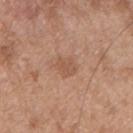{"biopsy_status": "not biopsied; imaged during a skin examination", "automated_metrics": {"area_mm2_approx": 4.0, "eccentricity": 0.6, "shape_asymmetry": 0.3, "cielab_L": 54, "cielab_a": 21, "cielab_b": 31, "vs_skin_darker_L": 7.0, "vs_skin_contrast_norm": 5.5, "color_variation_0_10": 2.0, "peripheral_color_asymmetry": 1.0, "nevus_likeness_0_100": 5, "lesion_detection_confidence_0_100": 100}, "lesion_size": {"long_diameter_mm_approx": 2.5}, "site": "chest", "patient": {"sex": "male", "age_approx": 55}, "image": {"source": "total-body photography crop", "field_of_view_mm": 15}}